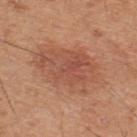follow-up: no biopsy performed (imaged during a skin exam); automated metrics: border irregularity of about 3.5 on a 0–10 scale and internal color variation of about 4.5 on a 0–10 scale; patient: male, roughly 45 years of age; lighting: white-light; imaging modality: ~15 mm crop, total-body skin-cancer survey; body site: the upper back; size: about 8 mm.